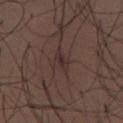Impression: Imaged during a routine full-body skin examination; the lesion was not biopsied and no histopathology is available. Background: Longest diameter approximately 2.5 mm. Located on the abdomen. The subject is a male aged approximately 30. A 15 mm crop from a total-body photograph taken for skin-cancer surveillance. The tile uses white-light illumination.